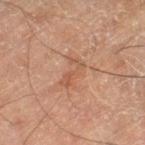The lesion was tiled from a total-body skin photograph and was not biopsied.